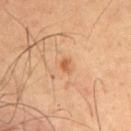Impression:
No biopsy was performed on this lesion — it was imaged during a full skin examination and was not determined to be concerning.
Image and clinical context:
Measured at roughly 2 mm in maximum diameter. Captured under cross-polarized illumination. The total-body-photography lesion software estimated a classifier nevus-likeness of about 50/100. The subject is a male about 65 years old. On the mid back. Cropped from a whole-body photographic skin survey; the tile spans about 15 mm.